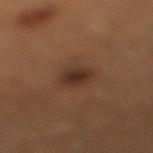Captured during whole-body skin photography for melanoma surveillance; the lesion was not biopsied. Cropped from a total-body skin-imaging series; the visible field is about 15 mm. Automated image analysis of the tile measured an area of roughly 6 mm² and a symmetry-axis asymmetry near 0.2. And it measured border irregularity of about 2 on a 0–10 scale, internal color variation of about 3.5 on a 0–10 scale, and peripheral color asymmetry of about 1. The analysis additionally found a nevus-likeness score of about 100/100. A male patient aged around 40. From the right lower leg.